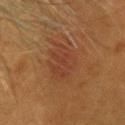Part of a total-body skin-imaging series; this lesion was reviewed on a skin check and was not flagged for biopsy. The lesion is on the head or neck. A female subject approximately 40 years of age. This is a cross-polarized tile. The lesion's longest dimension is about 6 mm. A lesion tile, about 15 mm wide, cut from a 3D total-body photograph. The total-body-photography lesion software estimated a footprint of about 13 mm², an eccentricity of roughly 0.85, and a symmetry-axis asymmetry near 0.25. And it measured a border-irregularity rating of about 3.5/10, a color-variation rating of about 3/10, and radial color variation of about 1.